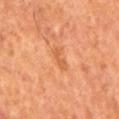<case>
<lighting>cross-polarized</lighting>
<lesion_size>
  <long_diameter_mm_approx>3.0</long_diameter_mm_approx>
</lesion_size>
<image>
  <source>total-body photography crop</source>
  <field_of_view_mm>15</field_of_view_mm>
</image>
<patient>
  <sex>male</sex>
  <age_approx>65</age_approx>
</patient>
</case>